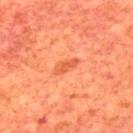<lesion>
<biopsy_status>not biopsied; imaged during a skin examination</biopsy_status>
<patient>
  <sex>male</sex>
  <age_approx>65</age_approx>
</patient>
<site>upper back</site>
<lesion_size>
  <long_diameter_mm_approx>3.0</long_diameter_mm_approx>
</lesion_size>
<image>
  <source>total-body photography crop</source>
  <field_of_view_mm>15</field_of_view_mm>
</image>
</lesion>